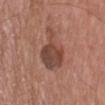| field | value |
|---|---|
| workup | catalogued during a skin exam; not biopsied |
| subject | male, aged around 55 |
| imaging modality | ~15 mm tile from a whole-body skin photo |
| anatomic site | the head or neck |
| automated lesion analysis | an eccentricity of roughly 0.65 and two-axis asymmetry of about 0.5; an automated nevus-likeness rating near 25 out of 100 and a detector confidence of about 100 out of 100 that the crop contains a lesion |